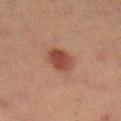Clinical summary:
Automated tile analysis of the lesion measured a footprint of about 7.5 mm² and a shape eccentricity near 0.75. The software also gave an average lesion color of about L≈36 a*≈21 b*≈24 (CIELAB) and a lesion–skin lightness drop of about 10. The software also gave a border-irregularity rating of about 2/10 and a within-lesion color-variation index near 3.5/10. Located on the right lower leg. Longest diameter approximately 4 mm. A roughly 15 mm field-of-view crop from a total-body skin photograph. A female patient aged around 55.